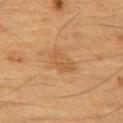Acquisition and patient details:
Automated tile analysis of the lesion measured a footprint of about 8 mm² and a symmetry-axis asymmetry near 0.3. The analysis additionally found a border-irregularity index near 3.5/10 and a peripheral color-asymmetry measure near 1. It also reported a nevus-likeness score of about 0/100. Cropped from a total-body skin-imaging series; the visible field is about 15 mm. Located on the left thigh. The recorded lesion diameter is about 4 mm. Captured under cross-polarized illumination. The subject is a male approximately 85 years of age or older.